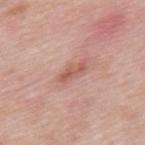Findings:
- notes · imaged on a skin check; not biopsied
- site · the mid back
- patient · male, aged around 55
- diameter · ≈3.5 mm
- acquisition · ~15 mm crop, total-body skin-cancer survey
- lighting · white-light